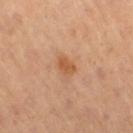The lesion was tiled from a total-body skin photograph and was not biopsied.
The lesion-visualizer software estimated a footprint of about 4 mm² and an eccentricity of roughly 0.75. And it measured border irregularity of about 2 on a 0–10 scale and a color-variation rating of about 1.5/10. And it measured a nevus-likeness score of about 45/100.
Captured under cross-polarized illumination.
The subject is a female aged 38 to 42.
Cropped from a whole-body photographic skin survey; the tile spans about 15 mm.
From the right thigh.
The recorded lesion diameter is about 2.5 mm.